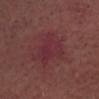workup=total-body-photography surveillance lesion; no biopsy.